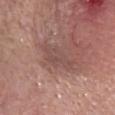Part of a total-body skin-imaging series; this lesion was reviewed on a skin check and was not flagged for biopsy. A 15 mm close-up extracted from a 3D total-body photography capture. Located on the head or neck. Approximately 4.5 mm at its widest. Captured under white-light illumination. A female subject aged around 65. The lesion-visualizer software estimated border irregularity of about 4.5 on a 0–10 scale, a color-variation rating of about 2/10, and a peripheral color-asymmetry measure near 0.5.